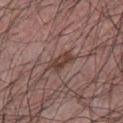biopsy_status: not biopsied; imaged during a skin examination
image:
  source: total-body photography crop
  field_of_view_mm: 15
patient:
  sex: male
  age_approx: 40
lesion_size:
  long_diameter_mm_approx: 3.0
site: chest
lighting: white-light